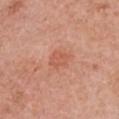site — the chest | patient — female, aged approximately 60 | acquisition — ~15 mm tile from a whole-body skin photo.